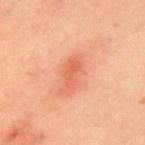The lesion was tiled from a total-body skin photograph and was not biopsied. A lesion tile, about 15 mm wide, cut from a 3D total-body photograph. Measured at roughly 4 mm in maximum diameter. A female patient aged around 40. Captured under cross-polarized illumination. From the upper back.